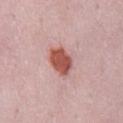{"biopsy_status": "not biopsied; imaged during a skin examination", "automated_metrics": {"cielab_L": 55, "cielab_a": 27, "cielab_b": 27, "vs_skin_darker_L": 15.0, "vs_skin_contrast_norm": 10.5, "border_irregularity_0_10": 2.0, "color_variation_0_10": 2.5, "peripheral_color_asymmetry": 1.0, "nevus_likeness_0_100": 100, "lesion_detection_confidence_0_100": 100}, "patient": {"sex": "male", "age_approx": 55}, "image": {"source": "total-body photography crop", "field_of_view_mm": 15}, "lighting": "white-light", "site": "lower back", "lesion_size": {"long_diameter_mm_approx": 4.0}}